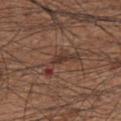Context: The patient is a male approximately 65 years of age. This is a white-light tile. Located on the abdomen. A roughly 15 mm field-of-view crop from a total-body skin photograph. Measured at roughly 5 mm in maximum diameter.